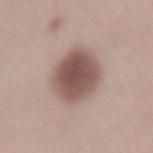| feature | finding |
|---|---|
| notes | imaged on a skin check; not biopsied |
| imaging modality | ~15 mm tile from a whole-body skin photo |
| patient | female, aged 23–27 |
| body site | the lower back |